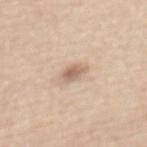Captured during whole-body skin photography for melanoma surveillance; the lesion was not biopsied. A female subject, in their mid-60s. The lesion's longest dimension is about 2.5 mm. The lesion is located on the mid back. This image is a 15 mm lesion crop taken from a total-body photograph.